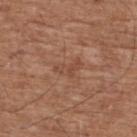| feature | finding |
|---|---|
| notes | no biopsy performed (imaged during a skin exam) |
| lesion size | ~3 mm (longest diameter) |
| location | the upper back |
| acquisition | ~15 mm tile from a whole-body skin photo |
| lighting | white-light illumination |
| automated metrics | a lesion area of about 3.5 mm², an eccentricity of roughly 0.85, and a shape-asymmetry score of about 0.55 (0 = symmetric); a classifier nevus-likeness of about 0/100 |
| subject | male, aged 73–77 |